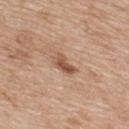{"lighting": "white-light", "patient": {"sex": "female", "age_approx": 40}, "automated_metrics": {"area_mm2_approx": 4.5, "eccentricity": 0.85, "nevus_likeness_0_100": 20, "lesion_detection_confidence_0_100": 100}, "image": {"source": "total-body photography crop", "field_of_view_mm": 15}, "site": "upper back"}